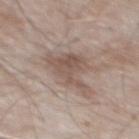Impression:
This lesion was catalogued during total-body skin photography and was not selected for biopsy.
Context:
Imaged with white-light lighting. The lesion is located on the mid back. An algorithmic analysis of the crop reported a border-irregularity index near 7/10 and a color-variation rating of about 3/10. The analysis additionally found an automated nevus-likeness rating near 15 out of 100. A male subject, aged 53–57. Approximately 6 mm at its widest. This image is a 15 mm lesion crop taken from a total-body photograph.